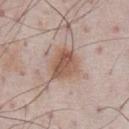Assessment:
Imaged during a routine full-body skin examination; the lesion was not biopsied and no histopathology is available.
Clinical summary:
The lesion-visualizer software estimated border irregularity of about 2 on a 0–10 scale, a within-lesion color-variation index near 5.5/10, and radial color variation of about 2. It also reported an automated nevus-likeness rating near 95 out of 100 and a detector confidence of about 100 out of 100 that the crop contains a lesion. The tile uses white-light illumination. Cropped from a total-body skin-imaging series; the visible field is about 15 mm. The subject is a male aged 68 to 72. On the chest.